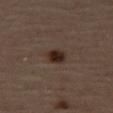workup — catalogued during a skin exam; not biopsied
lesion diameter — about 2.5 mm
acquisition — ~15 mm tile from a whole-body skin photo
illumination — cross-polarized illumination
patient — male, approximately 65 years of age
site — the leg